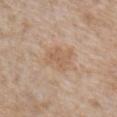biopsy status = no biopsy performed (imaged during a skin exam) | imaging modality = ~15 mm crop, total-body skin-cancer survey | lesion diameter = ≈3.5 mm | lighting = white-light illumination | patient = male, approximately 65 years of age | anatomic site = the right upper arm.